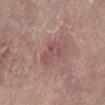The lesion was tiled from a total-body skin photograph and was not biopsied. A female patient roughly 65 years of age. A 15 mm close-up extracted from a 3D total-body photography capture. Approximately 3.5 mm at its widest. The tile uses white-light illumination. Located on the leg.